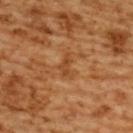Q: Is there a histopathology result?
A: no biopsy performed (imaged during a skin exam)
Q: Where on the body is the lesion?
A: the back
Q: Patient demographics?
A: female, in their mid- to late 50s
Q: How was this image acquired?
A: ~15 mm tile from a whole-body skin photo